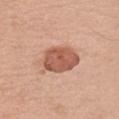biopsy status: no biopsy performed (imaged during a skin exam) | tile lighting: white-light | location: the chest | imaging modality: 15 mm crop, total-body photography | image-analysis metrics: a lesion color around L≈57 a*≈25 b*≈30 in CIELAB, a lesion–skin lightness drop of about 14, and a lesion-to-skin contrast of about 9 (normalized; higher = more distinct); a border-irregularity index near 2/10 and a within-lesion color-variation index near 3.5/10 | patient: male, in their mid- to late 50s.